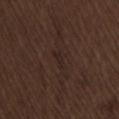Clinical summary:
The patient is a male aged approximately 70. This is a white-light tile. From the lower back. Cropped from a total-body skin-imaging series; the visible field is about 15 mm. The lesion-visualizer software estimated a lesion area of about 3 mm², a shape eccentricity near 0.9, and a shape-asymmetry score of about 0.3 (0 = symmetric). It also reported a border-irregularity index near 3.5/10 and a peripheral color-asymmetry measure near 0. The recorded lesion diameter is about 2.5 mm.